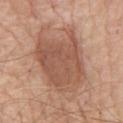– notes: no biopsy performed (imaged during a skin exam)
– subject: male, aged 78–82
– image source: 15 mm crop, total-body photography
– tile lighting: white-light
– lesion size: ~9 mm (longest diameter)
– location: the chest